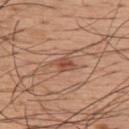Clinical impression:
No biopsy was performed on this lesion — it was imaged during a full skin examination and was not determined to be concerning.
Context:
The patient is a male aged 53 to 57. On the upper back. Cropped from a whole-body photographic skin survey; the tile spans about 15 mm.